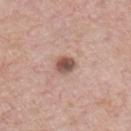Q: Was this lesion biopsied?
A: catalogued during a skin exam; not biopsied
Q: Where on the body is the lesion?
A: the chest
Q: What are the patient's age and sex?
A: male, about 55 years old
Q: What is the lesion's diameter?
A: about 3 mm
Q: Automated lesion metrics?
A: a border-irregularity rating of about 2/10, internal color variation of about 4.5 on a 0–10 scale, and a peripheral color-asymmetry measure near 1; an automated nevus-likeness rating near 85 out of 100 and lesion-presence confidence of about 100/100
Q: How was this image acquired?
A: ~15 mm tile from a whole-body skin photo